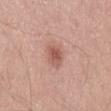Q: Was a biopsy performed?
A: no biopsy performed (imaged during a skin exam)
Q: What are the patient's age and sex?
A: male, aged 23 to 27
Q: What lighting was used for the tile?
A: white-light illumination
Q: How was this image acquired?
A: total-body-photography crop, ~15 mm field of view
Q: Where on the body is the lesion?
A: the lower back